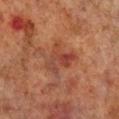  site: right lower leg
  image:
    source: total-body photography crop
    field_of_view_mm: 15
  patient:
    sex: male
    age_approx: 70
  automated_metrics:
    area_mm2_approx: 9.0
    eccentricity: 0.6
    nevus_likeness_0_100: 0
  lesion_size:
    long_diameter_mm_approx: 4.0